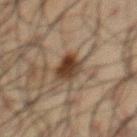A 15 mm close-up extracted from a 3D total-body photography capture.
The total-body-photography lesion software estimated a lesion area of about 6.5 mm², an outline eccentricity of about 0.65 (0 = round, 1 = elongated), and two-axis asymmetry of about 0.3. The analysis additionally found border irregularity of about 2.5 on a 0–10 scale and internal color variation of about 4.5 on a 0–10 scale.
A male patient, approximately 60 years of age.
The lesion is located on the front of the torso.
The lesion's longest dimension is about 3.5 mm.